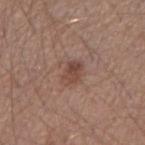Impression:
The lesion was tiled from a total-body skin photograph and was not biopsied.
Image and clinical context:
Longest diameter approximately 3 mm. Captured under white-light illumination. A lesion tile, about 15 mm wide, cut from a 3D total-body photograph. The lesion is located on the right upper arm. A male patient, approximately 55 years of age.